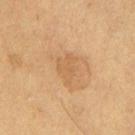workup — catalogued during a skin exam; not biopsied | image — total-body-photography crop, ~15 mm field of view | patient — female, roughly 70 years of age | illumination — cross-polarized | site — the right thigh.